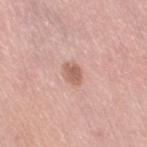{"biopsy_status": "not biopsied; imaged during a skin examination", "image": {"source": "total-body photography crop", "field_of_view_mm": 15}, "lesion_size": {"long_diameter_mm_approx": 2.5}, "site": "right thigh", "patient": {"sex": "female", "age_approx": 65}}